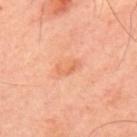{"biopsy_status": "not biopsied; imaged during a skin examination", "image": {"source": "total-body photography crop", "field_of_view_mm": 15}, "lighting": "cross-polarized", "site": "upper back", "patient": {"sex": "male", "age_approx": 50}, "lesion_size": {"long_diameter_mm_approx": 2.5}}